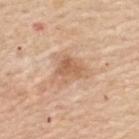A 15 mm close-up tile from a total-body photography series done for melanoma screening. The lesion is on the upper back. A female subject, in their mid- to late 60s. The total-body-photography lesion software estimated an area of roughly 7 mm², an eccentricity of roughly 0.6, and a symmetry-axis asymmetry near 0.35. The analysis additionally found a lesion color around L≈61 a*≈20 b*≈34 in CIELAB, roughly 10 lightness units darker than nearby skin, and a normalized lesion–skin contrast near 6.5. The analysis additionally found a nevus-likeness score of about 5/100. The lesion's longest dimension is about 3 mm. This is a white-light tile.